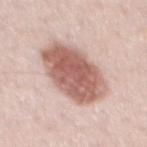Notes:
* follow-up · catalogued during a skin exam; not biopsied
* lighting · white-light illumination
* image-analysis metrics · a mean CIELAB color near L≈60 a*≈22 b*≈25 and a lesion–skin lightness drop of about 17; an automated nevus-likeness rating near 100 out of 100 and lesion-presence confidence of about 100/100
* imaging modality · total-body-photography crop, ~15 mm field of view
* subject · male, aged around 40
* diameter · ~7 mm (longest diameter)
* location · the mid back